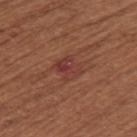The lesion was tiled from a total-body skin photograph and was not biopsied. Automated tile analysis of the lesion measured a footprint of about 6.5 mm² and an eccentricity of roughly 0.65. It also reported an average lesion color of about L≈39 a*≈26 b*≈26 (CIELAB) and a lesion-to-skin contrast of about 6 (normalized; higher = more distinct). The lesion is located on the upper back. Captured under white-light illumination. Cropped from a whole-body photographic skin survey; the tile spans about 15 mm. About 3.5 mm across. A female patient, roughly 65 years of age.